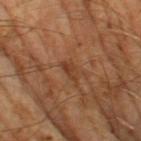{
  "biopsy_status": "not biopsied; imaged during a skin examination",
  "image": {
    "source": "total-body photography crop",
    "field_of_view_mm": 15
  },
  "lighting": "cross-polarized",
  "automated_metrics": {
    "area_mm2_approx": 3.0,
    "eccentricity": 0.85,
    "shape_asymmetry": 0.35,
    "border_irregularity_0_10": 3.5,
    "peripheral_color_asymmetry": 0.5
  },
  "patient": {
    "sex": "male",
    "age_approx": 65
  },
  "lesion_size": {
    "long_diameter_mm_approx": 2.5
  }
}